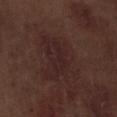automated lesion analysis: an area of roughly 4 mm², an eccentricity of roughly 0.9, and two-axis asymmetry of about 0.65; a mean CIELAB color near L≈22 a*≈18 b*≈16, roughly 4 lightness units darker than nearby skin, and a lesion-to-skin contrast of about 6 (normalized; higher = more distinct); a border-irregularity index near 7.5/10, a color-variation rating of about 1/10, and radial color variation of about 0.5; a nevus-likeness score of about 0/100 and lesion-presence confidence of about 90/100 | imaging modality: ~15 mm crop, total-body skin-cancer survey | illumination: white-light | subject: male, about 70 years old | site: the right lower leg | diameter: about 4 mm.